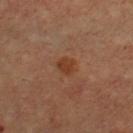Captured during whole-body skin photography for melanoma surveillance; the lesion was not biopsied.
A male subject approximately 65 years of age.
From the upper back.
A 15 mm close-up tile from a total-body photography series done for melanoma screening.